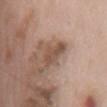Notes:
– notes: total-body-photography surveillance lesion; no biopsy
– diameter: ≈4 mm
– body site: the mid back
– patient: male, aged 63 to 67
– tile lighting: white-light illumination
– acquisition: ~15 mm crop, total-body skin-cancer survey